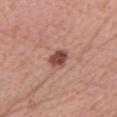biopsy status: total-body-photography surveillance lesion; no biopsy | location: the front of the torso | illumination: white-light illumination | subject: female, in their 60s | diameter: about 2.5 mm | acquisition: ~15 mm tile from a whole-body skin photo.